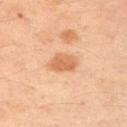Assessment:
Imaged during a routine full-body skin examination; the lesion was not biopsied and no histopathology is available.
Context:
Located on the left upper arm. An algorithmic analysis of the crop reported an area of roughly 6.5 mm² and a shape eccentricity near 0.75. The software also gave a lesion color around L≈64 a*≈24 b*≈39 in CIELAB and roughly 11 lightness units darker than nearby skin. The software also gave border irregularity of about 2.5 on a 0–10 scale, a within-lesion color-variation index near 2/10, and a peripheral color-asymmetry measure near 1. The analysis additionally found an automated nevus-likeness rating near 20 out of 100 and lesion-presence confidence of about 100/100. The subject is a male aged around 50. Captured under cross-polarized illumination. A region of skin cropped from a whole-body photographic capture, roughly 15 mm wide.